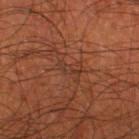{
  "biopsy_status": "not biopsied; imaged during a skin examination",
  "image": {
    "source": "total-body photography crop",
    "field_of_view_mm": 15
  },
  "patient": {
    "sex": "male",
    "age_approx": 60
  },
  "automated_metrics": {
    "area_mm2_approx": 2.5,
    "eccentricity": 0.95,
    "shape_asymmetry": 0.4
  },
  "lesion_size": {
    "long_diameter_mm_approx": 3.0
  },
  "site": "right thigh"
}